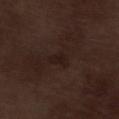Case summary:
- biopsy status — total-body-photography surveillance lesion; no biopsy